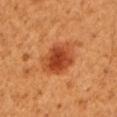Imaged during a routine full-body skin examination; the lesion was not biopsied and no histopathology is available.
Measured at roughly 5.5 mm in maximum diameter.
The total-body-photography lesion software estimated a within-lesion color-variation index near 5.5/10 and radial color variation of about 1.5.
The tile uses cross-polarized illumination.
This image is a 15 mm lesion crop taken from a total-body photograph.
The lesion is on the arm.
A male subject, aged 48 to 52.